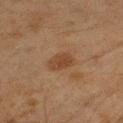Case summary:
• biopsy status · catalogued during a skin exam; not biopsied
• acquisition · ~15 mm crop, total-body skin-cancer survey
• patient · male, roughly 45 years of age
• anatomic site · the right upper arm